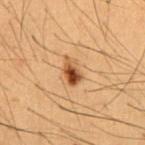No biopsy was performed on this lesion — it was imaged during a full skin examination and was not determined to be concerning. Cropped from a whole-body photographic skin survey; the tile spans about 15 mm. The patient is a male aged 48–52. The lesion is on the chest.